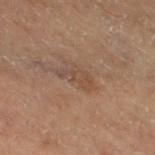site: the right thigh | lighting: cross-polarized | lesion diameter: ~3.5 mm (longest diameter) | patient: male, approximately 70 years of age | image source: total-body-photography crop, ~15 mm field of view.